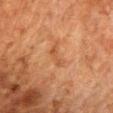Q: Was a biopsy performed?
A: no biopsy performed (imaged during a skin exam)
Q: Where on the body is the lesion?
A: the chest
Q: How was this image acquired?
A: ~15 mm crop, total-body skin-cancer survey
Q: How large is the lesion?
A: ≈3.5 mm
Q: Patient demographics?
A: male, about 80 years old